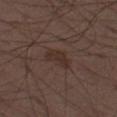Assessment:
This lesion was catalogued during total-body skin photography and was not selected for biopsy.
Image and clinical context:
A male subject roughly 50 years of age. The lesion is on the left thigh. Longest diameter approximately 3.5 mm. A 15 mm crop from a total-body photograph taken for skin-cancer surveillance.